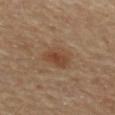Context:
Located on the mid back. This image is a 15 mm lesion crop taken from a total-body photograph. The tile uses cross-polarized illumination. The lesion-visualizer software estimated a mean CIELAB color near L≈42 a*≈18 b*≈30, roughly 8 lightness units darker than nearby skin, and a normalized border contrast of about 7. A male subject, approximately 85 years of age or older. About 4 mm across.